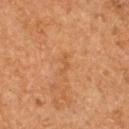notes: catalogued during a skin exam; not biopsied
automated metrics: a footprint of about 2 mm², an eccentricity of roughly 0.95, and a shape-asymmetry score of about 0.7 (0 = symmetric); a border-irregularity rating of about 7/10 and peripheral color asymmetry of about 0; a lesion-detection confidence of about 100/100
tile lighting: cross-polarized illumination
image source: ~15 mm crop, total-body skin-cancer survey
patient: female, about 60 years old
site: the upper back
size: about 2.5 mm